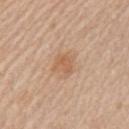Clinical impression:
Part of a total-body skin-imaging series; this lesion was reviewed on a skin check and was not flagged for biopsy.
Clinical summary:
Automated image analysis of the tile measured a lesion area of about 5 mm², an outline eccentricity of about 0.5 (0 = round, 1 = elongated), and two-axis asymmetry of about 0.3. The software also gave about 8 CIELAB-L* units darker than the surrounding skin and a lesion-to-skin contrast of about 6 (normalized; higher = more distinct). The lesion is located on the left upper arm. A close-up tile cropped from a whole-body skin photograph, about 15 mm across. A male subject aged approximately 75. Imaged with white-light lighting. About 2.5 mm across.